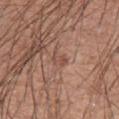Impression: Part of a total-body skin-imaging series; this lesion was reviewed on a skin check and was not flagged for biopsy. Context: This is a white-light tile. Longest diameter approximately 2.5 mm. This image is a 15 mm lesion crop taken from a total-body photograph. A male patient, roughly 60 years of age. The lesion is located on the abdomen. The lesion-visualizer software estimated a footprint of about 3 mm², an outline eccentricity of about 0.8 (0 = round, 1 = elongated), and a shape-asymmetry score of about 0.4 (0 = symmetric). The analysis additionally found a border-irregularity index near 4.5/10 and peripheral color asymmetry of about 0.5. The analysis additionally found an automated nevus-likeness rating near 5 out of 100.